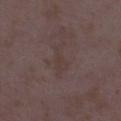biopsy status: no biopsy performed (imaged during a skin exam); subject: female, approximately 35 years of age; site: the leg; acquisition: 15 mm crop, total-body photography.